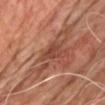Context:
A male subject, about 65 years old. Automated image analysis of the tile measured a footprint of about 4.5 mm² and a shape-asymmetry score of about 0.35 (0 = symmetric). The software also gave a mean CIELAB color near L≈42 a*≈24 b*≈28, roughly 8 lightness units darker than nearby skin, and a normalized lesion–skin contrast near 6. The software also gave a border-irregularity index near 3.5/10, a within-lesion color-variation index near 1.5/10, and peripheral color asymmetry of about 0.5. About 2.5 mm across. A 15 mm close-up tile from a total-body photography series done for melanoma screening. On the chest. This is a cross-polarized tile.